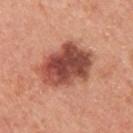Notes:
– biopsy status · total-body-photography surveillance lesion; no biopsy
– site · the upper back
– illumination · white-light
– automated lesion analysis · a border-irregularity index near 2.5/10, internal color variation of about 7.5 on a 0–10 scale, and radial color variation of about 2.5
– image · total-body-photography crop, ~15 mm field of view
– lesion diameter · ~7 mm (longest diameter)
– patient · male, aged 43–47